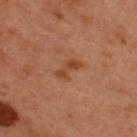Imaged during a routine full-body skin examination; the lesion was not biopsied and no histopathology is available.
Captured under cross-polarized illumination.
A male patient, aged 48–52.
Measured at roughly 3 mm in maximum diameter.
Cropped from a whole-body photographic skin survey; the tile spans about 15 mm.
The lesion-visualizer software estimated a footprint of about 4 mm² and a shape-asymmetry score of about 0.45 (0 = symmetric). And it measured an average lesion color of about L≈44 a*≈25 b*≈36 (CIELAB) and a normalized lesion–skin contrast near 7.5.
On the upper back.